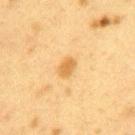Part of a total-body skin-imaging series; this lesion was reviewed on a skin check and was not flagged for biopsy. About 3 mm across. Automated tile analysis of the lesion measured a lesion area of about 4 mm², an eccentricity of roughly 0.8, and a shape-asymmetry score of about 0.25 (0 = symmetric). A 15 mm crop from a total-body photograph taken for skin-cancer surveillance. A female subject roughly 40 years of age. This is a cross-polarized tile. On the back.